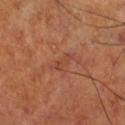<tbp_lesion>
<biopsy_status>not biopsied; imaged during a skin examination</biopsy_status>
<automated_metrics>
  <area_mm2_approx>2.5</area_mm2_approx>
  <eccentricity>0.9</eccentricity>
  <shape_asymmetry>0.4</shape_asymmetry>
  <border_irregularity_0_10>4.0</border_irregularity_0_10>
  <color_variation_0_10>0.0</color_variation_0_10>
  <peripheral_color_asymmetry>0.0</peripheral_color_asymmetry>
  <nevus_likeness_0_100>0</nevus_likeness_0_100>
  <lesion_detection_confidence_0_100>100</lesion_detection_confidence_0_100>
</automated_metrics>
<site>leg</site>
<lighting>cross-polarized</lighting>
<patient>
  <sex>male</sex>
  <age_approx>70</age_approx>
</patient>
<lesion_size>
  <long_diameter_mm_approx>2.5</long_diameter_mm_approx>
</lesion_size>
<image>
  <source>total-body photography crop</source>
  <field_of_view_mm>15</field_of_view_mm>
</image>
</tbp_lesion>